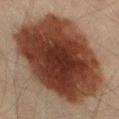Findings:
* biopsy status · imaged on a skin check; not biopsied
* anatomic site · the left thigh
* diameter · about 12.5 mm
* patient · male, aged approximately 40
* tile lighting · cross-polarized illumination
* image · 15 mm crop, total-body photography